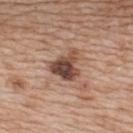{
  "biopsy_status": "not biopsied; imaged during a skin examination",
  "automated_metrics": {
    "border_irregularity_0_10": 5.0,
    "color_variation_0_10": 6.5,
    "peripheral_color_asymmetry": 2.0
  },
  "lesion_size": {
    "long_diameter_mm_approx": 4.0
  },
  "lighting": "white-light",
  "site": "upper back",
  "patient": {
    "sex": "female",
    "age_approx": 40
  },
  "image": {
    "source": "total-body photography crop",
    "field_of_view_mm": 15
  }
}